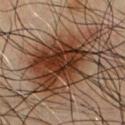Impression: The lesion was photographed on a routine skin check and not biopsied; there is no pathology result.